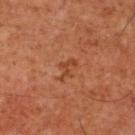{"biopsy_status": "not biopsied; imaged during a skin examination", "image": {"source": "total-body photography crop", "field_of_view_mm": 15}, "patient": {"sex": "male", "age_approx": 60}, "lighting": "cross-polarized", "lesion_size": {"long_diameter_mm_approx": 2.5}, "automated_metrics": {"area_mm2_approx": 2.5, "eccentricity": 0.85, "shape_asymmetry": 0.8, "color_variation_0_10": 0.0, "peripheral_color_asymmetry": 0.0, "nevus_likeness_0_100": 0, "lesion_detection_confidence_0_100": 100}, "site": "chest"}